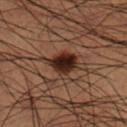Recorded during total-body skin imaging; not selected for excision or biopsy. From the right lower leg. The patient is a male about 50 years old. Measured at roughly 3.5 mm in maximum diameter. A region of skin cropped from a whole-body photographic capture, roughly 15 mm wide. The tile uses cross-polarized illumination.